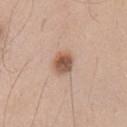Part of a total-body skin-imaging series; this lesion was reviewed on a skin check and was not flagged for biopsy.
Measured at roughly 3 mm in maximum diameter.
Automated tile analysis of the lesion measured internal color variation of about 5.5 on a 0–10 scale.
This is a white-light tile.
A male patient roughly 45 years of age.
A 15 mm crop from a total-body photograph taken for skin-cancer surveillance.
The lesion is located on the left upper arm.